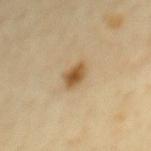Impression:
Imaged during a routine full-body skin examination; the lesion was not biopsied and no histopathology is available.
Background:
A male patient in their mid- to late 60s. A close-up tile cropped from a whole-body skin photograph, about 15 mm across. On the chest.